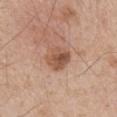| key | value |
|---|---|
| follow-up | imaged on a skin check; not biopsied |
| lighting | white-light illumination |
| body site | the mid back |
| subject | male, in their mid- to late 40s |
| size | about 3.5 mm |
| acquisition | ~15 mm tile from a whole-body skin photo |